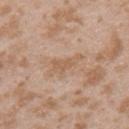Acquisition and patient details: The patient is a female aged 23 to 27. The lesion is on the arm. Imaged with white-light lighting. Cropped from a total-body skin-imaging series; the visible field is about 15 mm.